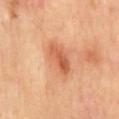The lesion was tiled from a total-body skin photograph and was not biopsied. Longest diameter approximately 5 mm. Captured under cross-polarized illumination. A region of skin cropped from a whole-body photographic capture, roughly 15 mm wide. On the mid back.